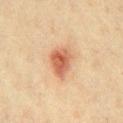The lesion is on the front of the torso. This image is a 15 mm lesion crop taken from a total-body photograph. The patient is a male aged approximately 40.